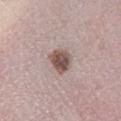<lesion>
<biopsy_status>not biopsied; imaged during a skin examination</biopsy_status>
<image>
  <source>total-body photography crop</source>
  <field_of_view_mm>15</field_of_view_mm>
</image>
<lesion_size>
  <long_diameter_mm_approx>3.0</long_diameter_mm_approx>
</lesion_size>
<patient>
  <sex>male</sex>
  <age_approx>50</age_approx>
</patient>
<site>right lower leg</site>
</lesion>